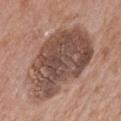The lesion is on the back. A 15 mm close-up extracted from a 3D total-body photography capture. This is a white-light tile. A male subject, aged around 65.